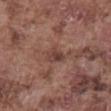This lesion was catalogued during total-body skin photography and was not selected for biopsy. The lesion is located on the abdomen. A male subject, roughly 75 years of age. This is a white-light tile. A 15 mm close-up extracted from a 3D total-body photography capture.